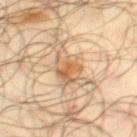A 15 mm close-up extracted from a 3D total-body photography capture. A male subject, aged around 65. From the right thigh. About 6.5 mm across. An algorithmic analysis of the crop reported a normalized border contrast of about 7. It also reported an automated nevus-likeness rating near 0 out of 100 and lesion-presence confidence of about 75/100.